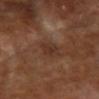Q: Was this lesion biopsied?
A: imaged on a skin check; not biopsied
Q: Patient demographics?
A: female, aged around 75
Q: What is the imaging modality?
A: ~15 mm tile from a whole-body skin photo
Q: What did automated image analysis measure?
A: a lesion area of about 5 mm², a shape eccentricity near 0.8, and a shape-asymmetry score of about 0.25 (0 = symmetric); about 6 CIELAB-L* units darker than the surrounding skin
Q: Lesion location?
A: the head or neck